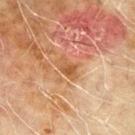Clinical impression: The lesion was tiled from a total-body skin photograph and was not biopsied. Context: Measured at roughly 3 mm in maximum diameter. A male patient, aged approximately 70. This image is a 15 mm lesion crop taken from a total-body photograph. Located on the left upper arm. An algorithmic analysis of the crop reported an average lesion color of about L≈46 a*≈20 b*≈33 (CIELAB) and roughly 7 lightness units darker than nearby skin.